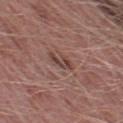  biopsy_status: not biopsied; imaged during a skin examination
  automated_metrics:
    border_irregularity_0_10: 5.5
    color_variation_0_10: 5.5
    peripheral_color_asymmetry: 2.5
    nevus_likeness_0_100: 0
    lesion_detection_confidence_0_100: 95
  image:
    source: total-body photography crop
    field_of_view_mm: 15
  lesion_size:
    long_diameter_mm_approx: 4.0
  patient:
    sex: male
    age_approx: 50
  site: left thigh
  lighting: white-light